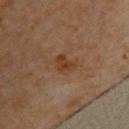Q: Was this lesion biopsied?
A: no biopsy performed (imaged during a skin exam)
Q: How was the tile lit?
A: cross-polarized
Q: What is the anatomic site?
A: the back
Q: Automated lesion metrics?
A: a mean CIELAB color near L≈38 a*≈19 b*≈31, a lesion–skin lightness drop of about 7, and a normalized border contrast of about 7; a border-irregularity rating of about 3.5/10 and peripheral color asymmetry of about 1
Q: What are the patient's age and sex?
A: female, aged approximately 65
Q: What kind of image is this?
A: 15 mm crop, total-body photography